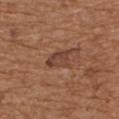Background:
An algorithmic analysis of the crop reported an average lesion color of about L≈42 a*≈22 b*≈28 (CIELAB), a lesion–skin lightness drop of about 9, and a lesion-to-skin contrast of about 7 (normalized; higher = more distinct). And it measured a border-irregularity index near 3.5/10 and radial color variation of about 1. It also reported an automated nevus-likeness rating near 0 out of 100 and a detector confidence of about 95 out of 100 that the crop contains a lesion. A male subject aged around 70. Captured under white-light illumination. On the upper back. The lesion's longest dimension is about 3.5 mm. This image is a 15 mm lesion crop taken from a total-body photograph.